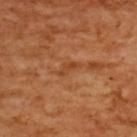Notes:
* biopsy status: no biopsy performed (imaged during a skin exam)
* lesion size: ~3 mm (longest diameter)
* TBP lesion metrics: a lesion area of about 2.5 mm², a shape eccentricity near 0.9, and a shape-asymmetry score of about 0.55 (0 = symmetric); a lesion-detection confidence of about 95/100
* patient: female, about 55 years old
* imaging modality: ~15 mm tile from a whole-body skin photo
* tile lighting: cross-polarized
* anatomic site: the upper back